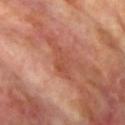Case summary:
* follow-up: no biopsy performed (imaged during a skin exam)
* patient: female, in their mid-70s
* site: the left upper arm
* image: ~15 mm tile from a whole-body skin photo
* illumination: cross-polarized
* diameter: ≈4 mm